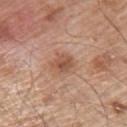follow-up: total-body-photography surveillance lesion; no biopsy | lesion diameter: ~3 mm (longest diameter) | location: the back | subject: male, roughly 55 years of age | tile lighting: white-light illumination | image: total-body-photography crop, ~15 mm field of view.